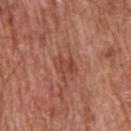workup: no biopsy performed (imaged during a skin exam) | patient: male, aged around 65 | image: ~15 mm tile from a whole-body skin photo | automated metrics: a lesion area of about 4.5 mm² and a shape eccentricity near 0.65 | tile lighting: white-light illumination | lesion diameter: ~2.5 mm (longest diameter) | location: the upper back.